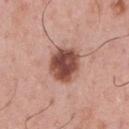A male patient in their 60s.
The tile uses white-light illumination.
A roughly 15 mm field-of-view crop from a total-body skin photograph.
On the front of the torso.